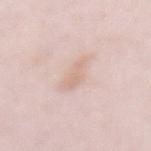Part of a total-body skin-imaging series; this lesion was reviewed on a skin check and was not flagged for biopsy.
The patient is a female approximately 65 years of age.
Automated image analysis of the tile measured an area of roughly 6.5 mm² and an eccentricity of roughly 0.9. The software also gave a border-irregularity index near 3.5/10 and a within-lesion color-variation index near 2/10. The analysis additionally found a classifier nevus-likeness of about 0/100.
A 15 mm close-up tile from a total-body photography series done for melanoma screening.
On the abdomen.
Captured under white-light illumination.
Longest diameter approximately 4.5 mm.